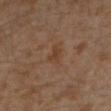Imaged during a routine full-body skin examination; the lesion was not biopsied and no histopathology is available. A male patient, roughly 30 years of age. A lesion tile, about 15 mm wide, cut from a 3D total-body photograph. Located on the left lower leg. The tile uses cross-polarized illumination.